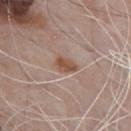Imaged during a routine full-body skin examination; the lesion was not biopsied and no histopathology is available.
Cropped from a whole-body photographic skin survey; the tile spans about 15 mm.
The recorded lesion diameter is about 3 mm.
On the chest.
Imaged with white-light lighting.
The lesion-visualizer software estimated a border-irregularity rating of about 2/10 and radial color variation of about 1. The analysis additionally found a detector confidence of about 100 out of 100 that the crop contains a lesion.
A male patient approximately 70 years of age.